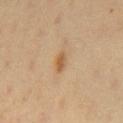Clinical impression:
Recorded during total-body skin imaging; not selected for excision or biopsy.
Image and clinical context:
Located on the chest. The lesion's longest dimension is about 2.5 mm. Captured under cross-polarized illumination. Cropped from a whole-body photographic skin survey; the tile spans about 15 mm. Automated tile analysis of the lesion measured an area of roughly 3 mm² and an outline eccentricity of about 0.85 (0 = round, 1 = elongated). A male subject, about 40 years old.